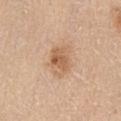Assessment:
Imaged during a routine full-body skin examination; the lesion was not biopsied and no histopathology is available.
Background:
A female patient aged around 65. On the front of the torso. This is a white-light tile. A 15 mm close-up tile from a total-body photography series done for melanoma screening.